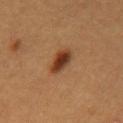This lesion was catalogued during total-body skin photography and was not selected for biopsy.
The lesion is located on the back.
A male subject, approximately 60 years of age.
Cropped from a whole-body photographic skin survey; the tile spans about 15 mm.